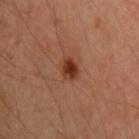A male patient aged 43 to 47.
Cropped from a whole-body photographic skin survey; the tile spans about 15 mm.
About 2.5 mm across.
From the left upper arm.
Imaged with cross-polarized lighting.